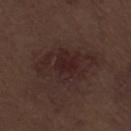• biopsy status · no biopsy performed (imaged during a skin exam)
• automated metrics · a lesion-to-skin contrast of about 7 (normalized; higher = more distinct); a border-irregularity index near 3/10 and internal color variation of about 3.5 on a 0–10 scale; a nevus-likeness score of about 35/100 and a detector confidence of about 100 out of 100 that the crop contains a lesion
• site · the right thigh
• image · ~15 mm tile from a whole-body skin photo
• patient · male, roughly 70 years of age